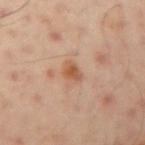Q: Is there a histopathology result?
A: imaged on a skin check; not biopsied
Q: What is the imaging modality?
A: 15 mm crop, total-body photography
Q: What are the patient's age and sex?
A: male, approximately 50 years of age
Q: How large is the lesion?
A: ~2.5 mm (longest diameter)
Q: What is the anatomic site?
A: the arm
Q: What lighting was used for the tile?
A: cross-polarized
Q: Automated lesion metrics?
A: a footprint of about 4 mm², an outline eccentricity of about 0.6 (0 = round, 1 = elongated), and a symmetry-axis asymmetry near 0.25; border irregularity of about 2 on a 0–10 scale, a within-lesion color-variation index near 4/10, and peripheral color asymmetry of about 1.5; a classifier nevus-likeness of about 5/100 and a detector confidence of about 100 out of 100 that the crop contains a lesion